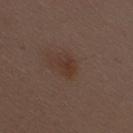No biopsy was performed on this lesion — it was imaged during a full skin examination and was not determined to be concerning. On the lower back. About 2.5 mm across. Automated tile analysis of the lesion measured a shape eccentricity near 0.7. And it measured a lesion–skin lightness drop of about 6 and a lesion-to-skin contrast of about 6 (normalized; higher = more distinct). It also reported a border-irregularity rating of about 4/10 and a within-lesion color-variation index near 1/10. A female patient, aged around 30. Cropped from a whole-body photographic skin survey; the tile spans about 15 mm.